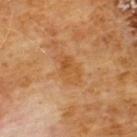A 15 mm close-up extracted from a 3D total-body photography capture.
Measured at roughly 4 mm in maximum diameter.
A male subject, in their 60s.
Automated image analysis of the tile measured a lesion area of about 6.5 mm², an outline eccentricity of about 0.85 (0 = round, 1 = elongated), and two-axis asymmetry of about 0.25. The software also gave a border-irregularity index near 3/10, a within-lesion color-variation index near 4.5/10, and a peripheral color-asymmetry measure near 1.5. The analysis additionally found a nevus-likeness score of about 0/100 and a lesion-detection confidence of about 100/100.
Imaged with cross-polarized lighting.
The lesion is on the chest.